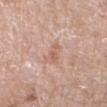Captured during whole-body skin photography for melanoma surveillance; the lesion was not biopsied. The tile uses white-light illumination. On the right forearm. A female patient, about 55 years old. The total-body-photography lesion software estimated a lesion color around L≈60 a*≈21 b*≈30 in CIELAB and a normalized border contrast of about 5.5. The software also gave a peripheral color-asymmetry measure near 0. And it measured a classifier nevus-likeness of about 0/100 and a detector confidence of about 100 out of 100 that the crop contains a lesion. Longest diameter approximately 3 mm. A 15 mm close-up extracted from a 3D total-body photography capture.